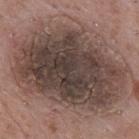notes — no biopsy performed (imaged during a skin exam) | location — the upper back | lighting — white-light | diameter — ~13.5 mm (longest diameter) | image source — 15 mm crop, total-body photography | patient — male, about 70 years old.